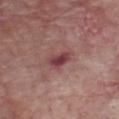follow-up: no biopsy performed (imaged during a skin exam)
illumination: white-light
automated lesion analysis: a border-irregularity index near 3/10, internal color variation of about 4.5 on a 0–10 scale, and a peripheral color-asymmetry measure near 1.5; a classifier nevus-likeness of about 0/100 and lesion-presence confidence of about 100/100
subject: male, aged approximately 65
body site: the chest
imaging modality: ~15 mm tile from a whole-body skin photo
size: ≈3 mm